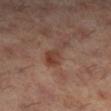Captured during whole-body skin photography for melanoma surveillance; the lesion was not biopsied.
A close-up tile cropped from a whole-body skin photograph, about 15 mm across.
The lesion is located on the leg.
This is a cross-polarized tile.
A female subject, aged 58–62.
About 5 mm across.